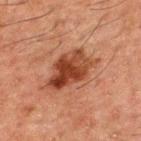Context:
A male patient in their 50s. The lesion is on the upper back. A roughly 15 mm field-of-view crop from a total-body skin photograph.